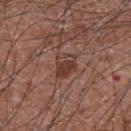Impression:
The lesion was photographed on a routine skin check and not biopsied; there is no pathology result.
Image and clinical context:
The recorded lesion diameter is about 3 mm. Located on the chest. A close-up tile cropped from a whole-body skin photograph, about 15 mm across. Automated image analysis of the tile measured an average lesion color of about L≈39 a*≈21 b*≈24 (CIELAB) and a normalized border contrast of about 7.5. The subject is a male about 75 years old.